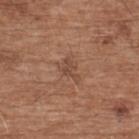Impression: Imaged during a routine full-body skin examination; the lesion was not biopsied and no histopathology is available. Image and clinical context: An algorithmic analysis of the crop reported a footprint of about 3.5 mm², an outline eccentricity of about 0.8 (0 = round, 1 = elongated), and two-axis asymmetry of about 0.5. The software also gave a lesion color around L≈46 a*≈20 b*≈29 in CIELAB, roughly 7 lightness units darker than nearby skin, and a normalized border contrast of about 5.5. The analysis additionally found border irregularity of about 6 on a 0–10 scale. Imaged with white-light lighting. A close-up tile cropped from a whole-body skin photograph, about 15 mm across. On the upper back. A male patient, about 75 years old.